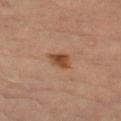{
  "biopsy_status": "not biopsied; imaged during a skin examination",
  "site": "left forearm",
  "patient": {
    "sex": "female",
    "age_approx": 65
  },
  "automated_metrics": {
    "cielab_L": 48,
    "cielab_a": 23,
    "cielab_b": 35,
    "vs_skin_darker_L": 12.0,
    "vs_skin_contrast_norm": 10.0
  },
  "lesion_size": {
    "long_diameter_mm_approx": 3.0
  },
  "lighting": "cross-polarized",
  "image": {
    "source": "total-body photography crop",
    "field_of_view_mm": 15
  }
}